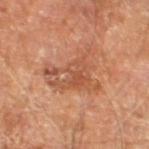notes = catalogued during a skin exam; not biopsied
lighting = cross-polarized illumination
imaging modality = total-body-photography crop, ~15 mm field of view
subject = male, aged 68 to 72
location = the leg
diameter = ~6 mm (longest diameter)